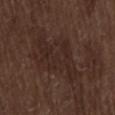Part of a total-body skin-imaging series; this lesion was reviewed on a skin check and was not flagged for biopsy. The tile uses white-light illumination. A male subject aged approximately 70. A region of skin cropped from a whole-body photographic capture, roughly 15 mm wide. The total-body-photography lesion software estimated a footprint of about 20 mm², an outline eccentricity of about 0.85 (0 = round, 1 = elongated), and two-axis asymmetry of about 0.35. The analysis additionally found an average lesion color of about L≈25 a*≈15 b*≈20 (CIELAB), roughly 5 lightness units darker than nearby skin, and a normalized border contrast of about 5.5. And it measured a border-irregularity rating of about 5/10 and internal color variation of about 2.5 on a 0–10 scale. The lesion is located on the lower back. About 7.5 mm across.